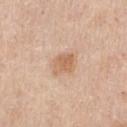Recorded during total-body skin imaging; not selected for excision or biopsy.
The lesion is on the chest.
Cropped from a total-body skin-imaging series; the visible field is about 15 mm.
The patient is a male in their 60s.
Measured at roughly 3 mm in maximum diameter.